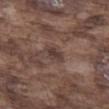| feature | finding |
|---|---|
| workup | total-body-photography surveillance lesion; no biopsy |
| acquisition | total-body-photography crop, ~15 mm field of view |
| patient | male, in their mid-70s |
| location | the left thigh |